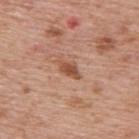workup — no biopsy performed (imaged during a skin exam) | size — ≈3 mm | location — the upper back | lighting — white-light | patient — male, in their mid-70s | automated metrics — a mean CIELAB color near L≈51 a*≈24 b*≈32, about 11 CIELAB-L* units darker than the surrounding skin, and a normalized lesion–skin contrast near 8; a within-lesion color-variation index near 2/10 and radial color variation of about 0.5; an automated nevus-likeness rating near 80 out of 100 | imaging modality — 15 mm crop, total-body photography.